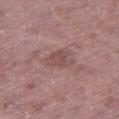workup = no biopsy performed (imaged during a skin exam); acquisition = 15 mm crop, total-body photography; patient = male, aged approximately 50; location = the right thigh; diameter = ~3.5 mm (longest diameter).